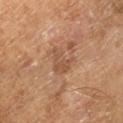Imaged during a routine full-body skin examination; the lesion was not biopsied and no histopathology is available. This image is a 15 mm lesion crop taken from a total-body photograph. The tile uses cross-polarized illumination. The lesion-visualizer software estimated a lesion area of about 6 mm², an eccentricity of roughly 0.55, and two-axis asymmetry of about 0.45. The analysis additionally found a border-irregularity rating of about 5/10, a within-lesion color-variation index near 2/10, and radial color variation of about 0.5. On the right lower leg. Longest diameter approximately 3.5 mm. The subject is a male aged 63–67.